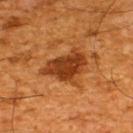No biopsy was performed on this lesion — it was imaged during a full skin examination and was not determined to be concerning. A close-up tile cropped from a whole-body skin photograph, about 15 mm across. Imaged with cross-polarized lighting. The lesion is on the upper back. The patient is a male in their mid- to late 60s. The lesion's longest dimension is about 5.5 mm.